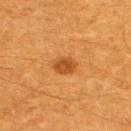biopsy status=total-body-photography surveillance lesion; no biopsy
site=the upper back
image=15 mm crop, total-body photography
diameter=≈2.5 mm
patient=male, aged around 60
illumination=cross-polarized illumination